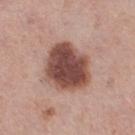follow-up: no biopsy performed (imaged during a skin exam)
size: about 5.5 mm
site: the right thigh
subject: female, roughly 40 years of age
automated metrics: a mean CIELAB color near L≈47 a*≈22 b*≈24 and a normalized border contrast of about 12.5; a border-irregularity rating of about 2/10, a color-variation rating of about 5/10, and radial color variation of about 1.5; a classifier nevus-likeness of about 55/100
image: total-body-photography crop, ~15 mm field of view
illumination: white-light